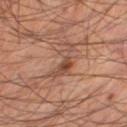Impression:
This lesion was catalogued during total-body skin photography and was not selected for biopsy.
Clinical summary:
The lesion is on the right thigh. The subject is a male aged 63 to 67. Cropped from a whole-body photographic skin survey; the tile spans about 15 mm.